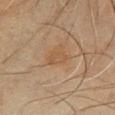Q: Is there a histopathology result?
A: no biopsy performed (imaged during a skin exam)
Q: Lesion location?
A: the left lower leg
Q: How large is the lesion?
A: ≈3 mm
Q: What did automated image analysis measure?
A: a border-irregularity rating of about 3/10, a color-variation rating of about 1/10, and radial color variation of about 0.5; a classifier nevus-likeness of about 0/100
Q: What is the imaging modality?
A: 15 mm crop, total-body photography
Q: Patient demographics?
A: male, roughly 65 years of age
Q: How was the tile lit?
A: cross-polarized illumination